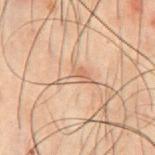Imaged during a routine full-body skin examination; the lesion was not biopsied and no histopathology is available.
A male patient, aged 48–52.
This is a cross-polarized tile.
The lesion's longest dimension is about 3.5 mm.
A region of skin cropped from a whole-body photographic capture, roughly 15 mm wide.
An algorithmic analysis of the crop reported a footprint of about 4 mm². It also reported peripheral color asymmetry of about 1. And it measured a classifier nevus-likeness of about 0/100 and a lesion-detection confidence of about 90/100.
From the chest.